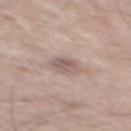Captured during whole-body skin photography for melanoma surveillance; the lesion was not biopsied. Captured under white-light illumination. A male subject, aged approximately 70. A roughly 15 mm field-of-view crop from a total-body skin photograph. Longest diameter approximately 3 mm. The lesion is on the upper back.